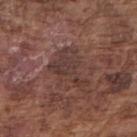Q: Is there a histopathology result?
A: catalogued during a skin exam; not biopsied
Q: Who is the patient?
A: male, roughly 75 years of age
Q: How large is the lesion?
A: ≈5.5 mm
Q: What is the anatomic site?
A: the right upper arm
Q: How was this image acquired?
A: 15 mm crop, total-body photography